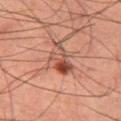Assessment: Captured during whole-body skin photography for melanoma surveillance; the lesion was not biopsied. Acquisition and patient details: The patient is a male aged approximately 60. Automated image analysis of the tile measured two-axis asymmetry of about 0.35. It also reported an average lesion color of about L≈39 a*≈20 b*≈23 (CIELAB) and a lesion-to-skin contrast of about 7.5 (normalized; higher = more distinct). The software also gave an automated nevus-likeness rating near 100 out of 100 and lesion-presence confidence of about 100/100. From the right thigh. A lesion tile, about 15 mm wide, cut from a 3D total-body photograph. This is a cross-polarized tile.